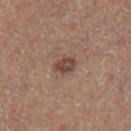Findings:
- notes: catalogued during a skin exam; not biopsied
- patient: male, approximately 65 years of age
- tile lighting: white-light illumination
- image source: ~15 mm crop, total-body skin-cancer survey
- image-analysis metrics: a lesion area of about 4.5 mm² and two-axis asymmetry of about 0.25; a border-irregularity index near 2/10, internal color variation of about 2.5 on a 0–10 scale, and a peripheral color-asymmetry measure near 1; a classifier nevus-likeness of about 35/100 and a detector confidence of about 100 out of 100 that the crop contains a lesion
- lesion size: ≈2.5 mm
- site: the left lower leg